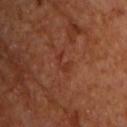workup = catalogued during a skin exam; not biopsied
image = 15 mm crop, total-body photography
patient = male, in their mid- to late 60s
lesion size = about 2.5 mm
tile lighting = cross-polarized
location = the chest